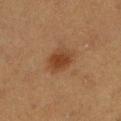Q: Is there a histopathology result?
A: no biopsy performed (imaged during a skin exam)
Q: What is the imaging modality?
A: ~15 mm tile from a whole-body skin photo
Q: What is the anatomic site?
A: the left lower leg
Q: What did automated image analysis measure?
A: border irregularity of about 2 on a 0–10 scale
Q: Who is the patient?
A: female, approximately 40 years of age
Q: How large is the lesion?
A: about 3.5 mm
Q: Illumination type?
A: cross-polarized